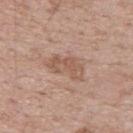Imaged during a routine full-body skin examination; the lesion was not biopsied and no histopathology is available.
Automated tile analysis of the lesion measured an area of roughly 8.5 mm² and two-axis asymmetry of about 0.35. And it measured a lesion color around L≈56 a*≈19 b*≈28 in CIELAB, about 8 CIELAB-L* units darker than the surrounding skin, and a normalized lesion–skin contrast near 6. And it measured an automated nevus-likeness rating near 0 out of 100 and lesion-presence confidence of about 100/100.
The lesion is on the back.
This is a white-light tile.
The lesion's longest dimension is about 4.5 mm.
A region of skin cropped from a whole-body photographic capture, roughly 15 mm wide.
A male subject aged 68–72.